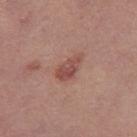Q: Was this lesion biopsied?
A: catalogued during a skin exam; not biopsied
Q: What are the patient's age and sex?
A: female, approximately 40 years of age
Q: What lighting was used for the tile?
A: white-light
Q: What is the lesion's diameter?
A: ~4 mm (longest diameter)
Q: What kind of image is this?
A: ~15 mm crop, total-body skin-cancer survey
Q: What is the anatomic site?
A: the right thigh